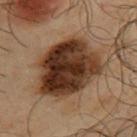{
  "patient": {
    "sex": "male",
    "age_approx": 65
  },
  "automated_metrics": {
    "border_irregularity_0_10": 2.0,
    "color_variation_0_10": 8.5,
    "peripheral_color_asymmetry": 3.0,
    "nevus_likeness_0_100": 65
  },
  "site": "upper back",
  "image": {
    "source": "total-body photography crop",
    "field_of_view_mm": 15
  },
  "lighting": "cross-polarized"
}